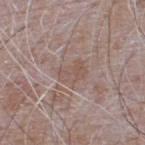Captured during whole-body skin photography for melanoma surveillance; the lesion was not biopsied. A male patient about 65 years old. Approximately 3.5 mm at its widest. From the upper back. This is a white-light tile. This image is a 15 mm lesion crop taken from a total-body photograph.